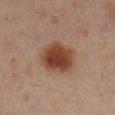Q: How was this image acquired?
A: ~15 mm tile from a whole-body skin photo
Q: Where on the body is the lesion?
A: the left leg
Q: Patient demographics?
A: female, aged 38–42